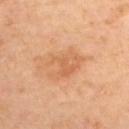{"biopsy_status": "not biopsied; imaged during a skin examination", "lighting": "cross-polarized", "patient": {"sex": "female", "age_approx": 45}, "automated_metrics": {"eccentricity": 0.65, "shape_asymmetry": 0.35, "cielab_L": 64, "cielab_a": 25, "cielab_b": 40, "vs_skin_darker_L": 7.0, "vs_skin_contrast_norm": 5.0, "border_irregularity_0_10": 5.5, "color_variation_0_10": 3.0, "peripheral_color_asymmetry": 1.0, "nevus_likeness_0_100": 0, "lesion_detection_confidence_0_100": 100}, "site": "upper back", "image": {"source": "total-body photography crop", "field_of_view_mm": 15}, "lesion_size": {"long_diameter_mm_approx": 4.5}}